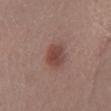Impression: No biopsy was performed on this lesion — it was imaged during a full skin examination and was not determined to be concerning. Background: On the right lower leg. Cropped from a total-body skin-imaging series; the visible field is about 15 mm. A male subject, in their 50s.